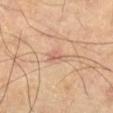Located on the right thigh. A 15 mm close-up tile from a total-body photography series done for melanoma screening. A male subject aged 68 to 72.